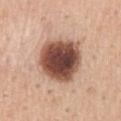No biopsy was performed on this lesion — it was imaged during a full skin examination and was not determined to be concerning.
Longest diameter approximately 5.5 mm.
A close-up tile cropped from a whole-body skin photograph, about 15 mm across.
Imaged with white-light lighting.
A male subject, in their 50s.
Automated tile analysis of the lesion measured a lesion color around L≈48 a*≈22 b*≈27 in CIELAB, a lesion–skin lightness drop of about 23, and a normalized lesion–skin contrast near 14.5. The software also gave a within-lesion color-variation index near 7.5/10 and peripheral color asymmetry of about 1.5.
Located on the mid back.